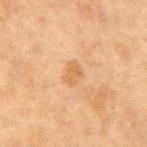Clinical impression:
The lesion was photographed on a routine skin check and not biopsied; there is no pathology result.
Background:
The recorded lesion diameter is about 3 mm. An algorithmic analysis of the crop reported a lesion color around L≈56 a*≈19 b*≈37 in CIELAB, about 7 CIELAB-L* units darker than the surrounding skin, and a lesion-to-skin contrast of about 5.5 (normalized; higher = more distinct). The software also gave a peripheral color-asymmetry measure near 0.5. And it measured an automated nevus-likeness rating near 0 out of 100. On the right upper arm. The patient is a female aged 38–42. This is a cross-polarized tile. A 15 mm close-up extracted from a 3D total-body photography capture.